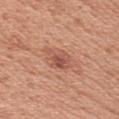Captured during whole-body skin photography for melanoma surveillance; the lesion was not biopsied.
A 15 mm close-up tile from a total-body photography series done for melanoma screening.
On the left upper arm.
Measured at roughly 3.5 mm in maximum diameter.
A female subject, in their 50s.